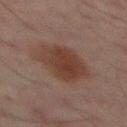No biopsy was performed on this lesion — it was imaged during a full skin examination and was not determined to be concerning. On the back. A male patient, in their mid-60s. The tile uses cross-polarized illumination. A region of skin cropped from a whole-body photographic capture, roughly 15 mm wide. An algorithmic analysis of the crop reported roughly 7 lightness units darker than nearby skin. The analysis additionally found a nevus-likeness score of about 90/100 and lesion-presence confidence of about 100/100.